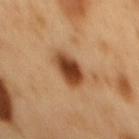follow-up: no biopsy performed (imaged during a skin exam) | lesion size: ≈4 mm | location: the mid back | patient: male, aged around 50 | automated metrics: about 17 CIELAB-L* units darker than the surrounding skin and a normalized lesion–skin contrast near 12.5 | imaging modality: ~15 mm tile from a whole-body skin photo | tile lighting: cross-polarized.